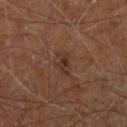biopsy status = total-body-photography surveillance lesion; no biopsy | site = the right leg | acquisition = ~15 mm tile from a whole-body skin photo | TBP lesion metrics = a lesion area of about 4 mm², a shape eccentricity near 0.8, and a symmetry-axis asymmetry near 0.4; an automated nevus-likeness rating near 0 out of 100 | illumination = cross-polarized | patient = male, roughly 60 years of age.